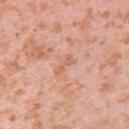Clinical impression:
Recorded during total-body skin imaging; not selected for excision or biopsy.
Image and clinical context:
A 15 mm crop from a total-body photograph taken for skin-cancer surveillance. The lesion is located on the upper back. A female subject, about 40 years old.